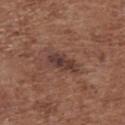Q: Is there a histopathology result?
A: total-body-photography surveillance lesion; no biopsy
Q: Who is the patient?
A: female, about 75 years old
Q: Lesion location?
A: the upper back
Q: How was this image acquired?
A: ~15 mm tile from a whole-body skin photo